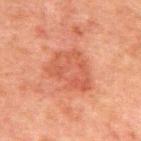image:
  source: total-body photography crop
  field_of_view_mm: 15
lesion_size:
  long_diameter_mm_approx: 5.5
patient:
  sex: male
  age_approx: 60
site: mid back
lighting: cross-polarized